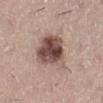Recorded during total-body skin imaging; not selected for excision or biopsy.
This is a white-light tile.
The patient is a female about 40 years old.
A region of skin cropped from a whole-body photographic capture, roughly 15 mm wide.
Located on the left lower leg.